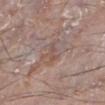Imaged during a routine full-body skin examination; the lesion was not biopsied and no histopathology is available. A male patient approximately 65 years of age. This image is a 15 mm lesion crop taken from a total-body photograph. Located on the left lower leg.